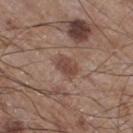Q: Was this lesion biopsied?
A: imaged on a skin check; not biopsied
Q: How large is the lesion?
A: about 2.5 mm
Q: What is the imaging modality?
A: 15 mm crop, total-body photography
Q: Patient demographics?
A: male, in their 60s
Q: What lighting was used for the tile?
A: white-light illumination
Q: Lesion location?
A: the right thigh